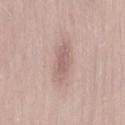| key | value |
|---|---|
| notes | total-body-photography surveillance lesion; no biopsy |